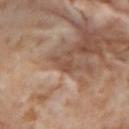Q: Is there a histopathology result?
A: imaged on a skin check; not biopsied
Q: Where on the body is the lesion?
A: the right thigh
Q: What are the patient's age and sex?
A: female, in their mid-50s
Q: What is the lesion's diameter?
A: ~2.5 mm (longest diameter)
Q: Automated lesion metrics?
A: a border-irregularity index near 5/10, a within-lesion color-variation index near 0.5/10, and radial color variation of about 0
Q: Illumination type?
A: cross-polarized
Q: What is the imaging modality?
A: ~15 mm crop, total-body skin-cancer survey